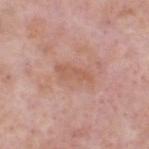<case>
<biopsy_status>not biopsied; imaged during a skin examination</biopsy_status>
<patient>
  <sex>male</sex>
  <age_approx>70</age_approx>
</patient>
<image>
  <source>total-body photography crop</source>
  <field_of_view_mm>15</field_of_view_mm>
</image>
<site>head or neck</site>
<lesion_size>
  <long_diameter_mm_approx>4.5</long_diameter_mm_approx>
</lesion_size>
<lighting>white-light</lighting>
</case>